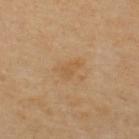Q: Was a biopsy performed?
A: no biopsy performed (imaged during a skin exam)
Q: What did automated image analysis measure?
A: a shape eccentricity near 0.85 and a symmetry-axis asymmetry near 0.25; a border-irregularity index near 2.5/10, a within-lesion color-variation index near 1/10, and a peripheral color-asymmetry measure near 0.5
Q: Patient demographics?
A: male, aged 53 to 57
Q: Lesion size?
A: about 2.5 mm
Q: Illumination type?
A: cross-polarized
Q: How was this image acquired?
A: ~15 mm tile from a whole-body skin photo
Q: Lesion location?
A: the upper back